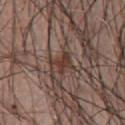Q: Was this lesion biopsied?
A: no biopsy performed (imaged during a skin exam)
Q: What lighting was used for the tile?
A: white-light illumination
Q: Patient demographics?
A: male, aged around 65
Q: What did automated image analysis measure?
A: a lesion–skin lightness drop of about 10 and a lesion-to-skin contrast of about 8.5 (normalized; higher = more distinct)
Q: Lesion location?
A: the chest
Q: What kind of image is this?
A: 15 mm crop, total-body photography